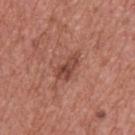biopsy_status: not biopsied; imaged during a skin examination
lesion_size:
  long_diameter_mm_approx: 3.5
automated_metrics:
  color_variation_0_10: 5.0
  nevus_likeness_0_100: 40
  lesion_detection_confidence_0_100: 100
site: upper back
image:
  source: total-body photography crop
  field_of_view_mm: 15
patient:
  sex: male
  age_approx: 70
lighting: white-light